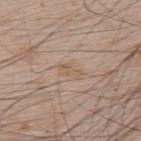Assessment:
No biopsy was performed on this lesion — it was imaged during a full skin examination and was not determined to be concerning.
Clinical summary:
A lesion tile, about 15 mm wide, cut from a 3D total-body photograph. The patient is a male aged around 65. Located on the upper back.